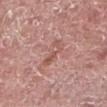biopsy_status: not biopsied; imaged during a skin examination
lesion_size:
  long_diameter_mm_approx: 3.5
image:
  source: total-body photography crop
  field_of_view_mm: 15
automated_metrics:
  border_irregularity_0_10: 6.5
  color_variation_0_10: 0.0
  peripheral_color_asymmetry: 0.0
patient:
  sex: male
  age_approx: 75
site: left lower leg